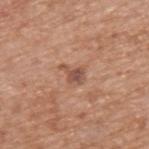<record>
<biopsy_status>not biopsied; imaged during a skin examination</biopsy_status>
<lighting>white-light</lighting>
<patient>
  <sex>male</sex>
  <age_approx>65</age_approx>
</patient>
<image>
  <source>total-body photography crop</source>
  <field_of_view_mm>15</field_of_view_mm>
</image>
<site>upper back</site>
<automated_metrics>
  <lesion_detection_confidence_0_100>100</lesion_detection_confidence_0_100>
</automated_metrics>
<lesion_size>
  <long_diameter_mm_approx>2.5</long_diameter_mm_approx>
</lesion_size>
</record>